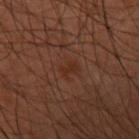{"biopsy_status": "not biopsied; imaged during a skin examination", "image": {"source": "total-body photography crop", "field_of_view_mm": 15}, "site": "right forearm", "lesion_size": {"long_diameter_mm_approx": 2.5}, "lighting": "cross-polarized", "patient": {"sex": "male", "age_approx": 60}}